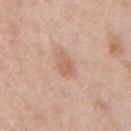The lesion is on the right upper arm. Cropped from a total-body skin-imaging series; the visible field is about 15 mm. The lesion-visualizer software estimated a footprint of about 4.5 mm², an eccentricity of roughly 0.7, and a symmetry-axis asymmetry near 0.25. And it measured an average lesion color of about L≈62 a*≈20 b*≈30 (CIELAB), about 8 CIELAB-L* units darker than the surrounding skin, and a normalized lesion–skin contrast near 6. It also reported border irregularity of about 2 on a 0–10 scale, a color-variation rating of about 2.5/10, and peripheral color asymmetry of about 1. A female subject about 50 years old. About 2.5 mm across.